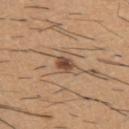| feature | finding |
|---|---|
| notes | imaged on a skin check; not biopsied |